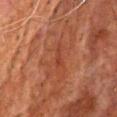The lesion was photographed on a routine skin check and not biopsied; there is no pathology result.
A male subject aged approximately 65.
A close-up tile cropped from a whole-body skin photograph, about 15 mm across.
The lesion is located on the chest.